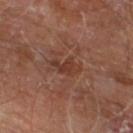Assessment: Recorded during total-body skin imaging; not selected for excision or biopsy. Context: The tile uses cross-polarized illumination. On the left lower leg. Cropped from a total-body skin-imaging series; the visible field is about 15 mm. The patient is a male aged approximately 70.